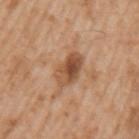Assessment: This lesion was catalogued during total-body skin photography and was not selected for biopsy. Acquisition and patient details: An algorithmic analysis of the crop reported a lesion color around L≈51 a*≈21 b*≈34 in CIELAB, about 12 CIELAB-L* units darker than the surrounding skin, and a lesion-to-skin contrast of about 8.5 (normalized; higher = more distinct). And it measured a border-irregularity rating of about 2/10, internal color variation of about 7.5 on a 0–10 scale, and peripheral color asymmetry of about 2.5. The software also gave an automated nevus-likeness rating near 50 out of 100 and a lesion-detection confidence of about 100/100. A 15 mm close-up extracted from a 3D total-body photography capture. The lesion is located on the left upper arm. The patient is a male aged approximately 65.